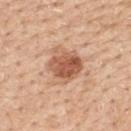Findings:
- biopsy status · catalogued during a skin exam; not biopsied
- tile lighting · white-light illumination
- image source · 15 mm crop, total-body photography
- TBP lesion metrics · a mean CIELAB color near L≈58 a*≈23 b*≈33, a lesion–skin lightness drop of about 13, and a normalized border contrast of about 8.5
- location · the upper back
- subject · male, approximately 60 years of age
- lesion diameter · about 4.5 mm